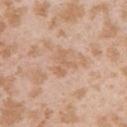biopsy_status: not biopsied; imaged during a skin examination
patient:
  sex: female
  age_approx: 25
lesion_size:
  long_diameter_mm_approx: 3.0
image:
  source: total-body photography crop
  field_of_view_mm: 15
site: arm
automated_metrics:
  shape_asymmetry: 0.55
  nevus_likeness_0_100: 0